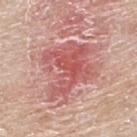Findings:
* biopsy status — no biopsy performed (imaged during a skin exam)
* anatomic site — the back
* lesion diameter — ≈7.5 mm
* image-analysis metrics — a mean CIELAB color near L≈57 a*≈30 b*≈25, roughly 11 lightness units darker than nearby skin, and a normalized lesion–skin contrast near 7
* tile lighting — white-light
* patient — male, aged 63 to 67
* imaging modality — total-body-photography crop, ~15 mm field of view